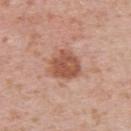Acquisition and patient details: A close-up tile cropped from a whole-body skin photograph, about 15 mm across. From the upper back. The subject is a male aged around 40. Imaged with white-light lighting. About 4 mm across.